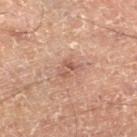Assessment: No biopsy was performed on this lesion — it was imaged during a full skin examination and was not determined to be concerning. Acquisition and patient details: A 15 mm close-up tile from a total-body photography series done for melanoma screening. A male patient aged around 50. The lesion is on the right lower leg. Approximately 3 mm at its widest.